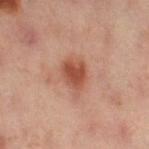Q: Was a biopsy performed?
A: catalogued during a skin exam; not biopsied
Q: What are the patient's age and sex?
A: female, aged around 40
Q: What did automated image analysis measure?
A: a footprint of about 8 mm², a shape eccentricity near 0.75, and two-axis asymmetry of about 0.35; a border-irregularity rating of about 3.5/10, a color-variation rating of about 3.5/10, and radial color variation of about 1; an automated nevus-likeness rating near 95 out of 100
Q: What lighting was used for the tile?
A: cross-polarized illumination
Q: Lesion size?
A: ~4 mm (longest diameter)
Q: What is the imaging modality?
A: ~15 mm tile from a whole-body skin photo
Q: Lesion location?
A: the right thigh